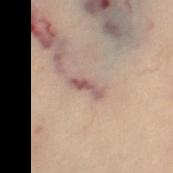A roughly 15 mm field-of-view crop from a total-body skin photograph.
The total-body-photography lesion software estimated an outline eccentricity of about 0.95 (0 = round, 1 = elongated) and a symmetry-axis asymmetry near 0.4. The software also gave an average lesion color of about L≈48 a*≈16 b*≈19 (CIELAB), roughly 9 lightness units darker than nearby skin, and a normalized lesion–skin contrast near 7.5. The analysis additionally found a border-irregularity index near 4.5/10 and a peripheral color-asymmetry measure near 0.
The subject is a female approximately 60 years of age.
This is a cross-polarized tile.
The recorded lesion diameter is about 3.5 mm.
The lesion is on the right leg.